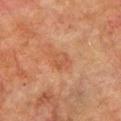This lesion was catalogued during total-body skin photography and was not selected for biopsy. Measured at roughly 2.5 mm in maximum diameter. Cropped from a whole-body photographic skin survey; the tile spans about 15 mm. A male patient, in their mid-70s. On the left upper arm. Captured under cross-polarized illumination.